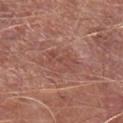{"biopsy_status": "not biopsied; imaged during a skin examination", "lesion_size": {"long_diameter_mm_approx": 4.5}, "site": "leg", "image": {"source": "total-body photography crop", "field_of_view_mm": 15}, "patient": {"sex": "male", "age_approx": 65}, "lighting": "white-light", "automated_metrics": {"eccentricity": 0.85, "shape_asymmetry": 0.35, "vs_skin_darker_L": 6.0, "vs_skin_contrast_norm": 4.5, "lesion_detection_confidence_0_100": 95}}